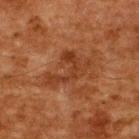Recorded during total-body skin imaging; not selected for excision or biopsy. A 15 mm close-up extracted from a 3D total-body photography capture. Located on the upper back. A male patient in their 60s. The tile uses cross-polarized illumination. Approximately 6 mm at its widest.